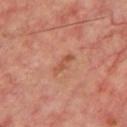Case summary:
• notes — catalogued during a skin exam; not biopsied
• image source — ~15 mm tile from a whole-body skin photo
• location — the chest
• lesion diameter — ~3 mm (longest diameter)
• subject — male, in their mid-60s
• tile lighting — cross-polarized